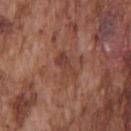<record>
  <biopsy_status>not biopsied; imaged during a skin examination</biopsy_status>
  <automated_metrics>
    <shape_asymmetry>0.35</shape_asymmetry>
    <border_irregularity_0_10>4.0</border_irregularity_0_10>
    <color_variation_0_10>3.5</color_variation_0_10>
    <peripheral_color_asymmetry>1.0</peripheral_color_asymmetry>
  </automated_metrics>
  <lesion_size>
    <long_diameter_mm_approx>3.5</long_diameter_mm_approx>
  </lesion_size>
  <lighting>white-light</lighting>
  <site>front of the torso</site>
  <image>
    <source>total-body photography crop</source>
    <field_of_view_mm>15</field_of_view_mm>
  </image>
  <patient>
    <sex>male</sex>
    <age_approx>75</age_approx>
  </patient>
</record>